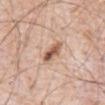  biopsy_status: not biopsied; imaged during a skin examination
  lesion_size:
    long_diameter_mm_approx: 3.0
  image:
    source: total-body photography crop
    field_of_view_mm: 15
  patient:
    sex: male
    age_approx: 80
  site: abdomen